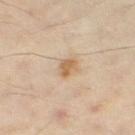Recorded during total-body skin imaging; not selected for excision or biopsy. The tile uses cross-polarized illumination. Approximately 2.5 mm at its widest. Located on the right thigh. A 15 mm crop from a total-body photograph taken for skin-cancer surveillance. The patient is a female aged around 45. An algorithmic analysis of the crop reported an average lesion color of about L≈63 a*≈17 b*≈36 (CIELAB), roughly 10 lightness units darker than nearby skin, and a lesion-to-skin contrast of about 7.5 (normalized; higher = more distinct).